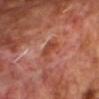biopsy status: no biopsy performed (imaged during a skin exam); image source: ~15 mm tile from a whole-body skin photo; patient: male, aged around 70; site: the chest.